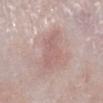{"biopsy_status": "not biopsied; imaged during a skin examination", "site": "left lower leg", "patient": {"sex": "male", "age_approx": 65}, "image": {"source": "total-body photography crop", "field_of_view_mm": 15}}